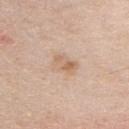Assessment:
The lesion was tiled from a total-body skin photograph and was not biopsied.
Context:
This is a white-light tile. On the chest. A 15 mm close-up extracted from a 3D total-body photography capture. The recorded lesion diameter is about 3.5 mm. A male patient about 45 years old.